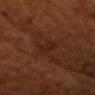A male patient roughly 60 years of age. An algorithmic analysis of the crop reported an area of roughly 5 mm² and a shape eccentricity near 0.65. The analysis additionally found border irregularity of about 2.5 on a 0–10 scale, internal color variation of about 2 on a 0–10 scale, and a peripheral color-asymmetry measure near 0.5. The lesion is located on the head or neck. This image is a 15 mm lesion crop taken from a total-body photograph.